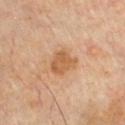| key | value |
|---|---|
| workup | catalogued during a skin exam; not biopsied |
| patient | male, about 60 years old |
| site | the chest |
| imaging modality | ~15 mm tile from a whole-body skin photo |